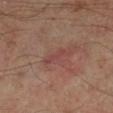notes — total-body-photography surveillance lesion; no biopsy | subject — male, aged 48–52 | image — ~15 mm tile from a whole-body skin photo | size — ~3.5 mm (longest diameter) | illumination — cross-polarized illumination | body site — the left leg.